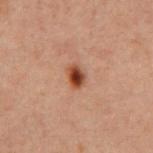Recorded during total-body skin imaging; not selected for excision or biopsy. About 2.5 mm across. From the chest. Cropped from a whole-body photographic skin survey; the tile spans about 15 mm. The subject is a male approximately 60 years of age.